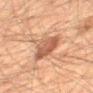workup: imaged on a skin check; not biopsied | size: about 5 mm | imaging modality: ~15 mm crop, total-body skin-cancer survey | lighting: cross-polarized | patient: male, about 60 years old | TBP lesion metrics: a lesion area of about 12 mm², a shape eccentricity near 0.7, and two-axis asymmetry of about 0.3; a lesion–skin lightness drop of about 10 and a lesion-to-skin contrast of about 8 (normalized; higher = more distinct); a border-irregularity index near 3.5/10 and a color-variation rating of about 4.5/10 | body site: the mid back.